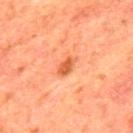workup: no biopsy performed (imaged during a skin exam); illumination: cross-polarized; location: the mid back; image source: ~15 mm tile from a whole-body skin photo; lesion size: ~2.5 mm (longest diameter); subject: male, approximately 65 years of age.